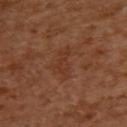biopsy_status: not biopsied; imaged during a skin examination
image:
  source: total-body photography crop
  field_of_view_mm: 15
lesion_size:
  long_diameter_mm_approx: 4.0
patient:
  sex: female
  age_approx: 55
site: upper back
lighting: cross-polarized